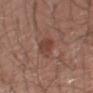Impression:
The lesion was photographed on a routine skin check and not biopsied; there is no pathology result.
Clinical summary:
A 15 mm crop from a total-body photograph taken for skin-cancer surveillance. A male subject, aged 48–52. On the right upper arm.